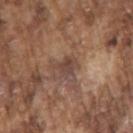Assessment:
Imaged during a routine full-body skin examination; the lesion was not biopsied and no histopathology is available.
Background:
The total-body-photography lesion software estimated a footprint of about 3.5 mm², an eccentricity of roughly 0.5, and a symmetry-axis asymmetry near 0.3. A 15 mm close-up extracted from a 3D total-body photography capture. Located on the right upper arm. A male patient, aged approximately 75. The tile uses white-light illumination. About 2.5 mm across.